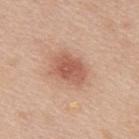Q: Is there a histopathology result?
A: no biopsy performed (imaged during a skin exam)
Q: Patient demographics?
A: male, aged 48–52
Q: What kind of image is this?
A: ~15 mm tile from a whole-body skin photo
Q: Lesion size?
A: ≈4 mm
Q: What is the anatomic site?
A: the mid back
Q: How was the tile lit?
A: white-light